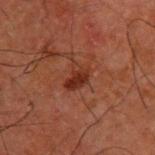<record>
  <biopsy_status>not biopsied; imaged during a skin examination</biopsy_status>
  <site>upper back</site>
  <automated_metrics>
    <area_mm2_approx>6.0</area_mm2_approx>
    <eccentricity>0.7</eccentricity>
    <cielab_L>24</cielab_L>
    <cielab_a>21</cielab_a>
    <cielab_b>24</cielab_b>
    <vs_skin_darker_L>7.0</vs_skin_darker_L>
    <vs_skin_contrast_norm>8.0</vs_skin_contrast_norm>
  </automated_metrics>
  <image>
    <source>total-body photography crop</source>
    <field_of_view_mm>15</field_of_view_mm>
  </image>
  <lesion_size>
    <long_diameter_mm_approx>3.0</long_diameter_mm_approx>
  </lesion_size>
  <patient>
    <sex>male</sex>
    <age_approx>50</age_approx>
  </patient>
</record>